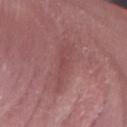Impression:
Imaged during a routine full-body skin examination; the lesion was not biopsied and no histopathology is available.
Context:
This is a white-light tile. The subject is a male aged around 60. An algorithmic analysis of the crop reported a lesion color around L≈47 a*≈25 b*≈20 in CIELAB, about 6 CIELAB-L* units darker than the surrounding skin, and a normalized border contrast of about 4.5. The lesion's longest dimension is about 5.5 mm. Located on the right forearm. A 15 mm crop from a total-body photograph taken for skin-cancer surveillance.